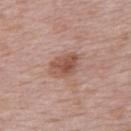follow-up: catalogued during a skin exam; not biopsied | lighting: white-light illumination | TBP lesion metrics: an outline eccentricity of about 0.7 (0 = round, 1 = elongated); border irregularity of about 2.5 on a 0–10 scale, a color-variation rating of about 4.5/10, and peripheral color asymmetry of about 1.5; a nevus-likeness score of about 70/100 and a detector confidence of about 100 out of 100 that the crop contains a lesion | subject: female, in their mid-60s | image source: 15 mm crop, total-body photography | location: the upper back | lesion size: ≈4 mm.